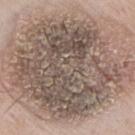biopsy_status: not biopsied; imaged during a skin examination
image:
  source: total-body photography crop
  field_of_view_mm: 15
site: right thigh
lesion_size:
  long_diameter_mm_approx: 14.5
patient:
  sex: male
  age_approx: 75
lighting: white-light